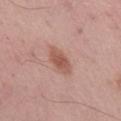This lesion was catalogued during total-body skin photography and was not selected for biopsy. From the mid back. A 15 mm crop from a total-body photograph taken for skin-cancer surveillance. Imaged with white-light lighting. An algorithmic analysis of the crop reported border irregularity of about 2 on a 0–10 scale, a within-lesion color-variation index near 2.5/10, and a peripheral color-asymmetry measure near 0.5. It also reported a classifier nevus-likeness of about 80/100 and a detector confidence of about 100 out of 100 that the crop contains a lesion. The subject is a male aged 53–57.